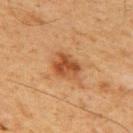Q: Was this lesion biopsied?
A: no biopsy performed (imaged during a skin exam)
Q: What did automated image analysis measure?
A: an average lesion color of about L≈41 a*≈23 b*≈34 (CIELAB), roughly 10 lightness units darker than nearby skin, and a normalized border contrast of about 8.5; border irregularity of about 2 on a 0–10 scale and a within-lesion color-variation index near 4/10; a nevus-likeness score of about 90/100
Q: What is the anatomic site?
A: the back
Q: How large is the lesion?
A: ≈3.5 mm
Q: What kind of image is this?
A: total-body-photography crop, ~15 mm field of view
Q: Who is the patient?
A: male, approximately 60 years of age